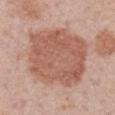Recorded during total-body skin imaging; not selected for excision or biopsy. On the right upper arm. The patient is a male aged 58 to 62. A roughly 15 mm field-of-view crop from a total-body skin photograph. The recorded lesion diameter is about 8.5 mm.